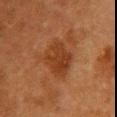Acquisition and patient details:
The subject is a female in their 50s. About 4 mm across. This is a cross-polarized tile. This image is a 15 mm lesion crop taken from a total-body photograph. From the chest. Automated image analysis of the tile measured a footprint of about 12 mm² and a symmetry-axis asymmetry near 0.2. And it measured an average lesion color of about L≈32 a*≈22 b*≈31 (CIELAB), roughly 7 lightness units darker than nearby skin, and a normalized border contrast of about 7.5.